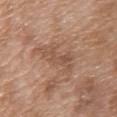  biopsy_status: not biopsied; imaged during a skin examination
  lesion_size:
    long_diameter_mm_approx: 5.5
  automated_metrics:
    border_irregularity_0_10: 7.5
    color_variation_0_10: 3.5
    peripheral_color_asymmetry: 1.0
    nevus_likeness_0_100: 0
    lesion_detection_confidence_0_100: 70
  image:
    source: total-body photography crop
    field_of_view_mm: 15
  patient:
    sex: female
    age_approx: 70
  site: upper back
  lighting: white-light